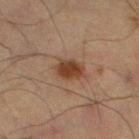This lesion was catalogued during total-body skin photography and was not selected for biopsy.
The lesion is located on the leg.
A 15 mm close-up tile from a total-body photography series done for melanoma screening.
A male subject aged 63 to 67.
Automated image analysis of the tile measured a footprint of about 6 mm², an outline eccentricity of about 0.6 (0 = round, 1 = elongated), and a symmetry-axis asymmetry near 0.15. The analysis additionally found a detector confidence of about 100 out of 100 that the crop contains a lesion.
The lesion's longest dimension is about 3 mm.